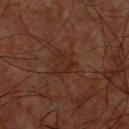The lesion was photographed on a routine skin check and not biopsied; there is no pathology result.
The subject is a male aged 58–62.
From the chest.
The recorded lesion diameter is about 3 mm.
A region of skin cropped from a whole-body photographic capture, roughly 15 mm wide.